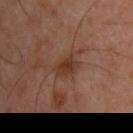Image and clinical context: Longest diameter approximately 3.5 mm. Located on the arm. Cropped from a whole-body photographic skin survey; the tile spans about 15 mm. A male subject about 45 years old.